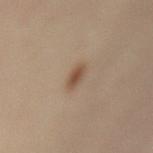Imaged during a routine full-body skin examination; the lesion was not biopsied and no histopathology is available. A female patient, about 40 years old. A lesion tile, about 15 mm wide, cut from a 3D total-body photograph. Located on the mid back.